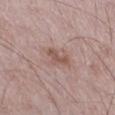Impression: No biopsy was performed on this lesion — it was imaged during a full skin examination and was not determined to be concerning. Context: A male subject aged 48–52. The tile uses white-light illumination. Automated image analysis of the tile measured a mean CIELAB color near L≈53 a*≈19 b*≈24, a lesion–skin lightness drop of about 9, and a lesion-to-skin contrast of about 6.5 (normalized; higher = more distinct). It also reported an automated nevus-likeness rating near 0 out of 100 and a detector confidence of about 100 out of 100 that the crop contains a lesion. This image is a 15 mm lesion crop taken from a total-body photograph. About 2.5 mm across. Located on the right thigh.